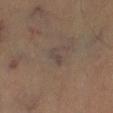workup: no biopsy performed (imaged during a skin exam)
image: 15 mm crop, total-body photography
subject: male, in their mid- to late 40s
location: the leg